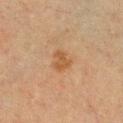This lesion was catalogued during total-body skin photography and was not selected for biopsy.
A 15 mm crop from a total-body photograph taken for skin-cancer surveillance.
The lesion is on the front of the torso.
The lesion-visualizer software estimated a lesion area of about 4 mm² and a shape eccentricity near 0.65. It also reported a mean CIELAB color near L≈43 a*≈18 b*≈32, about 7 CIELAB-L* units darker than the surrounding skin, and a normalized lesion–skin contrast near 7.
The lesion's longest dimension is about 2.5 mm.
The subject is a female aged 53 to 57.
The tile uses cross-polarized illumination.